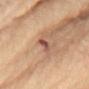Impression: Imaged during a routine full-body skin examination; the lesion was not biopsied and no histopathology is available. Context: An algorithmic analysis of the crop reported a lesion area of about 2.5 mm² and a shape eccentricity near 0.95. The software also gave border irregularity of about 4 on a 0–10 scale. Located on the left arm. A close-up tile cropped from a whole-body skin photograph, about 15 mm across. Approximately 3 mm at its widest. A female subject approximately 65 years of age.